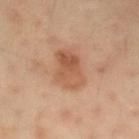Findings:
– imaging modality — ~15 mm tile from a whole-body skin photo
– TBP lesion metrics — a lesion area of about 11 mm², an eccentricity of roughly 0.85, and two-axis asymmetry of about 0.2; an average lesion color of about L≈54 a*≈22 b*≈33 (CIELAB), roughly 9 lightness units darker than nearby skin, and a normalized lesion–skin contrast near 6.5; a nevus-likeness score of about 35/100 and a lesion-detection confidence of about 100/100
– diameter — ~5 mm (longest diameter)
– anatomic site — the left forearm
– patient — male, aged 43–47